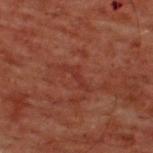Captured during whole-body skin photography for melanoma surveillance; the lesion was not biopsied. The total-body-photography lesion software estimated a border-irregularity rating of about 7/10, internal color variation of about 0 on a 0–10 scale, and radial color variation of about 0. The tile uses cross-polarized illumination. Cropped from a whole-body photographic skin survey; the tile spans about 15 mm. Measured at roughly 3.5 mm in maximum diameter. A male patient, aged around 60. The lesion is located on the upper back.